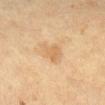<lesion>
  <biopsy_status>not biopsied; imaged during a skin examination</biopsy_status>
  <site>right lower leg</site>
  <lesion_size>
    <long_diameter_mm_approx>3.0</long_diameter_mm_approx>
  </lesion_size>
  <patient>
    <sex>female</sex>
    <age_approx>50</age_approx>
  </patient>
  <lighting>cross-polarized</lighting>
  <image>
    <source>total-body photography crop</source>
    <field_of_view_mm>15</field_of_view_mm>
  </image>
  <automated_metrics>
    <area_mm2_approx>6.0</area_mm2_approx>
    <eccentricity>0.35</eccentricity>
    <cielab_L>56</cielab_L>
    <cielab_a>15</cielab_a>
    <cielab_b>34</cielab_b>
    <nevus_likeness_0_100>0</nevus_likeness_0_100>
    <lesion_detection_confidence_0_100>100</lesion_detection_confidence_0_100>
  </automated_metrics>
</lesion>